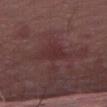biopsy status — imaged on a skin check; not biopsied
subject — male, aged around 75
lighting — white-light illumination
image — ~15 mm crop, total-body skin-cancer survey
anatomic site — the abdomen
diameter — ≈4.5 mm